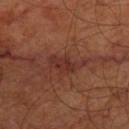Captured during whole-body skin photography for melanoma surveillance; the lesion was not biopsied. Imaged with cross-polarized lighting. The lesion is located on the right thigh. The patient is in their mid- to late 60s. A 15 mm close-up extracted from a 3D total-body photography capture.